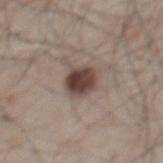Findings:
* workup — no biopsy performed (imaged during a skin exam)
* subject — male, approximately 50 years of age
* imaging modality — 15 mm crop, total-body photography
* lighting — white-light illumination
* image-analysis metrics — a lesion area of about 7.5 mm², an outline eccentricity of about 0.4 (0 = round, 1 = elongated), and a symmetry-axis asymmetry near 0.15; border irregularity of about 1.5 on a 0–10 scale and peripheral color asymmetry of about 2; a classifier nevus-likeness of about 95/100
* anatomic site — the mid back